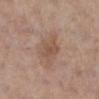notes — no biopsy performed (imaged during a skin exam) | subject — female, aged around 60 | automated metrics — a border-irregularity rating of about 2.5/10, a within-lesion color-variation index near 1.5/10, and radial color variation of about 0.5 | lesion diameter — about 3 mm | image source — total-body-photography crop, ~15 mm field of view | location — the right lower leg.